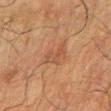Captured during whole-body skin photography for melanoma surveillance; the lesion was not biopsied. The tile uses cross-polarized illumination. Longest diameter approximately 3 mm. Located on the right forearm. A male patient in their 60s. Automated image analysis of the tile measured a lesion area of about 5.5 mm² and a shape eccentricity near 0.3. And it measured internal color variation of about 2.5 on a 0–10 scale and radial color variation of about 1. A 15 mm close-up extracted from a 3D total-body photography capture.